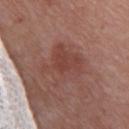{
  "biopsy_status": "not biopsied; imaged during a skin examination"
}